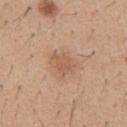Captured during whole-body skin photography for melanoma surveillance; the lesion was not biopsied. A male subject, in their 40s. The lesion is located on the front of the torso. Cropped from a total-body skin-imaging series; the visible field is about 15 mm. Approximately 3 mm at its widest. Imaged with white-light lighting.